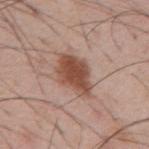Assessment:
No biopsy was performed on this lesion — it was imaged during a full skin examination and was not determined to be concerning.
Background:
A roughly 15 mm field-of-view crop from a total-body skin photograph. From the mid back. The tile uses white-light illumination. A male patient roughly 55 years of age. Longest diameter approximately 5.5 mm.